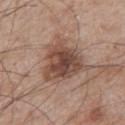This lesion was catalogued during total-body skin photography and was not selected for biopsy. A male patient aged 63 to 67. A roughly 15 mm field-of-view crop from a total-body skin photograph. Imaged with white-light lighting. The lesion is located on the left upper arm.